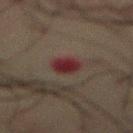– notes: imaged on a skin check; not biopsied
– acquisition: ~15 mm crop, total-body skin-cancer survey
– body site: the abdomen
– lighting: cross-polarized illumination
– patient: male, roughly 60 years of age
– automated metrics: a nevus-likeness score of about 0/100 and lesion-presence confidence of about 100/100